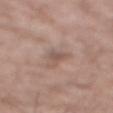workup: no biopsy performed (imaged during a skin exam); imaging modality: total-body-photography crop, ~15 mm field of view; site: the back; patient: male, in their mid- to late 40s; lighting: white-light illumination; size: ~3 mm (longest diameter).